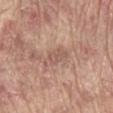{"biopsy_status": "not biopsied; imaged during a skin examination", "site": "mid back", "lesion_size": {"long_diameter_mm_approx": 4.0}, "image": {"source": "total-body photography crop", "field_of_view_mm": 15}, "automated_metrics": {"area_mm2_approx": 5.0, "eccentricity": 0.9, "shape_asymmetry": 0.3, "cielab_L": 56, "cielab_a": 19, "cielab_b": 26, "vs_skin_darker_L": 8.0, "vs_skin_contrast_norm": 5.5, "color_variation_0_10": 2.0, "peripheral_color_asymmetry": 1.0, "lesion_detection_confidence_0_100": 95}, "patient": {"sex": "male", "age_approx": 65}, "lighting": "white-light"}